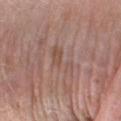Imaged during a routine full-body skin examination; the lesion was not biopsied and no histopathology is available. A male patient about 75 years old. An algorithmic analysis of the crop reported a lesion area of about 6.5 mm² and a symmetry-axis asymmetry near 0.45. It also reported an average lesion color of about L≈52 a*≈18 b*≈25 (CIELAB), about 6 CIELAB-L* units darker than the surrounding skin, and a normalized border contrast of about 4.5. The tile uses white-light illumination. From the right forearm. A 15 mm close-up tile from a total-body photography series done for melanoma screening. Longest diameter approximately 4 mm.